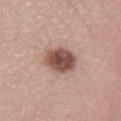A region of skin cropped from a whole-body photographic capture, roughly 15 mm wide.
The tile uses white-light illumination.
An algorithmic analysis of the crop reported a lesion color around L≈50 a*≈20 b*≈24 in CIELAB, about 16 CIELAB-L* units darker than the surrounding skin, and a normalized lesion–skin contrast near 11. The software also gave a within-lesion color-variation index near 5/10.
About 4 mm across.
From the right lower leg.
A female subject, approximately 30 years of age.